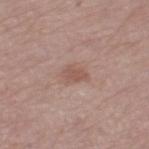workup: no biopsy performed (imaged during a skin exam) | subject: female, aged around 65 | diameter: about 2.5 mm | tile lighting: white-light illumination | image source: total-body-photography crop, ~15 mm field of view | site: the leg | image-analysis metrics: a footprint of about 3.5 mm² and a symmetry-axis asymmetry near 0.3; a lesion color around L≈53 a*≈20 b*≈24 in CIELAB, about 8 CIELAB-L* units darker than the surrounding skin, and a lesion-to-skin contrast of about 6 (normalized; higher = more distinct); an automated nevus-likeness rating near 10 out of 100 and a lesion-detection confidence of about 100/100.